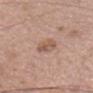Assessment: The lesion was tiled from a total-body skin photograph and was not biopsied. Acquisition and patient details: Located on the head or neck. A roughly 15 mm field-of-view crop from a total-body skin photograph. A female patient aged 33 to 37.